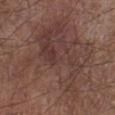Case summary:
* notes · total-body-photography surveillance lesion; no biopsy
* site · the leg
* lesion diameter · ≈12.5 mm
* image source · ~15 mm crop, total-body skin-cancer survey
* automated lesion analysis · an area of roughly 75 mm², an outline eccentricity of about 0.45 (0 = round, 1 = elongated), and two-axis asymmetry of about 0.45; radial color variation of about 1.5
* illumination · white-light illumination
* patient · male, approximately 55 years of age